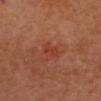| field | value |
|---|---|
| biopsy status | total-body-photography surveillance lesion; no biopsy |
| image | ~15 mm tile from a whole-body skin photo |
| body site | the head or neck |
| subject | female, approximately 55 years of age |